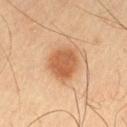Clinical summary: The patient is a male roughly 65 years of age. A 15 mm close-up extracted from a 3D total-body photography capture. From the left thigh. This is a cross-polarized tile. The lesion's longest dimension is about 4 mm. Automated tile analysis of the lesion measured an area of roughly 11 mm² and an eccentricity of roughly 0.55. The analysis additionally found a lesion color around L≈47 a*≈20 b*≈32 in CIELAB, a lesion–skin lightness drop of about 10, and a normalized lesion–skin contrast near 8. It also reported a border-irregularity rating of about 1.5/10, internal color variation of about 2.5 on a 0–10 scale, and peripheral color asymmetry of about 1.